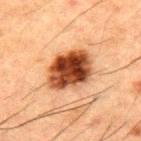Assessment:
Imaged during a routine full-body skin examination; the lesion was not biopsied and no histopathology is available.
Acquisition and patient details:
Imaged with cross-polarized lighting. A male patient aged 48–52. Located on the upper back. A lesion tile, about 15 mm wide, cut from a 3D total-body photograph. The recorded lesion diameter is about 5.5 mm. An algorithmic analysis of the crop reported a lesion–skin lightness drop of about 20 and a lesion-to-skin contrast of about 15 (normalized; higher = more distinct). And it measured a border-irregularity rating of about 1.5/10 and peripheral color asymmetry of about 2.5.